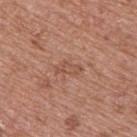workup: imaged on a skin check; not biopsied | image-analysis metrics: a footprint of about 4 mm², a shape eccentricity near 0.9, and a symmetry-axis asymmetry near 0.45; a border-irregularity index near 4.5/10 and internal color variation of about 1.5 on a 0–10 scale; lesion-presence confidence of about 100/100 | acquisition: ~15 mm crop, total-body skin-cancer survey | subject: male, in their mid- to late 50s | anatomic site: the upper back | tile lighting: white-light | lesion diameter: about 3.5 mm.